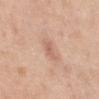Part of a total-body skin-imaging series; this lesion was reviewed on a skin check and was not flagged for biopsy.
The recorded lesion diameter is about 3 mm.
Captured under white-light illumination.
From the abdomen.
Cropped from a whole-body photographic skin survey; the tile spans about 15 mm.
A female subject aged around 40.